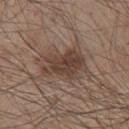Recorded during total-body skin imaging; not selected for excision or biopsy. The lesion is located on the chest. An algorithmic analysis of the crop reported an area of roughly 14 mm² and two-axis asymmetry of about 0.3. The subject is a male approximately 80 years of age. This is a white-light tile. Cropped from a total-body skin-imaging series; the visible field is about 15 mm.